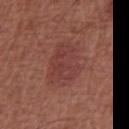{
  "biopsy_status": "not biopsied; imaged during a skin examination",
  "lesion_size": {
    "long_diameter_mm_approx": 5.5
  },
  "site": "abdomen",
  "lighting": "white-light",
  "patient": {
    "sex": "male",
    "age_approx": 65
  },
  "image": {
    "source": "total-body photography crop",
    "field_of_view_mm": 15
  }
}